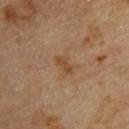Impression: The lesion was photographed on a routine skin check and not biopsied; there is no pathology result. Background: Longest diameter approximately 2.5 mm. A male patient about 85 years old or older. This is a cross-polarized tile. A lesion tile, about 15 mm wide, cut from a 3D total-body photograph. On the upper back.